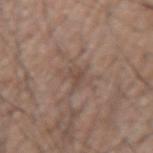The lesion was tiled from a total-body skin photograph and was not biopsied.
The tile uses white-light illumination.
The recorded lesion diameter is about 2.5 mm.
Automated tile analysis of the lesion measured an average lesion color of about L≈47 a*≈16 b*≈24 (CIELAB) and about 7 CIELAB-L* units darker than the surrounding skin. The software also gave internal color variation of about 3 on a 0–10 scale and a peripheral color-asymmetry measure near 1.
A male patient, in their 60s.
The lesion is on the right upper arm.
A 15 mm close-up extracted from a 3D total-body photography capture.